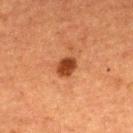Recorded during total-body skin imaging; not selected for excision or biopsy. The lesion is located on the back. This is a cross-polarized tile. A lesion tile, about 15 mm wide, cut from a 3D total-body photograph. A male subject in their 50s. The lesion-visualizer software estimated border irregularity of about 2 on a 0–10 scale and a within-lesion color-variation index near 2.5/10. The software also gave a classifier nevus-likeness of about 95/100 and a lesion-detection confidence of about 100/100.